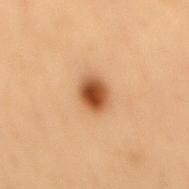workup: no biopsy performed (imaged during a skin exam) | lesion size: ≈3.5 mm | subject: male, about 55 years old | imaging modality: total-body-photography crop, ~15 mm field of view | location: the mid back | tile lighting: cross-polarized illumination.